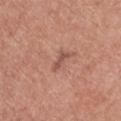Part of a total-body skin-imaging series; this lesion was reviewed on a skin check and was not flagged for biopsy.
A close-up tile cropped from a whole-body skin photograph, about 15 mm across.
The lesion is located on the upper back.
A female subject, roughly 60 years of age.